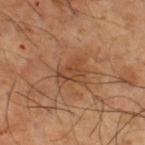This lesion was catalogued during total-body skin photography and was not selected for biopsy. About 3.5 mm across. The tile uses cross-polarized illumination. Located on the right thigh. A lesion tile, about 15 mm wide, cut from a 3D total-body photograph. A male subject, aged approximately 65.